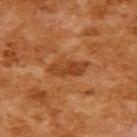biopsy status: imaged on a skin check; not biopsied
image: ~15 mm tile from a whole-body skin photo
automated metrics: a peripheral color-asymmetry measure near 1; a detector confidence of about 100 out of 100 that the crop contains a lesion
subject: female, aged approximately 55
site: the upper back
tile lighting: cross-polarized illumination
diameter: ~4.5 mm (longest diameter)